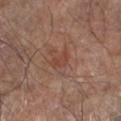| key | value |
|---|---|
| workup | total-body-photography surveillance lesion; no biopsy |
| image | ~15 mm crop, total-body skin-cancer survey |
| anatomic site | the left lower leg |
| diameter | about 2.5 mm |
| lighting | white-light illumination |
| subject | male, aged 68 to 72 |